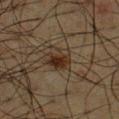A 15 mm close-up extracted from a 3D total-body photography capture. An algorithmic analysis of the crop reported an area of roughly 7 mm² and a shape-asymmetry score of about 0.35 (0 = symmetric). The analysis additionally found a lesion–skin lightness drop of about 8 and a normalized border contrast of about 9.5. The software also gave a border-irregularity rating of about 4/10 and a within-lesion color-variation index near 4/10. A male subject aged 58–62. Imaged with cross-polarized lighting. From the front of the torso.